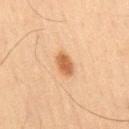Case summary:
• biopsy status: total-body-photography surveillance lesion; no biopsy
• anatomic site: the right thigh
• automated metrics: a mean CIELAB color near L≈49 a*≈20 b*≈34
• subject: male, aged around 65
• illumination: cross-polarized illumination
• image: total-body-photography crop, ~15 mm field of view